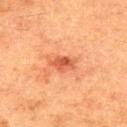notes=imaged on a skin check; not biopsied.